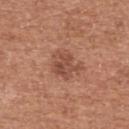| field | value |
|---|---|
| biopsy status | no biopsy performed (imaged during a skin exam) |
| anatomic site | the upper back |
| illumination | white-light illumination |
| acquisition | 15 mm crop, total-body photography |
| subject | female, about 60 years old |